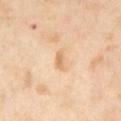Impression:
This lesion was catalogued during total-body skin photography and was not selected for biopsy.
Context:
The subject is a female aged 48 to 52. Approximately 2 mm at its widest. Cropped from a whole-body photographic skin survey; the tile spans about 15 mm. On the right thigh. Automated image analysis of the tile measured a footprint of about 2 mm², a shape eccentricity near 0.9, and a shape-asymmetry score of about 0.35 (0 = symmetric). The analysis additionally found an automated nevus-likeness rating near 20 out of 100.